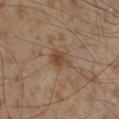notes: no biopsy performed (imaged during a skin exam)
tile lighting: cross-polarized
subject: male, aged approximately 55
body site: the right lower leg
diameter: about 3 mm
acquisition: 15 mm crop, total-body photography
automated metrics: a lesion color around L≈45 a*≈16 b*≈28 in CIELAB and a lesion–skin lightness drop of about 8; a classifier nevus-likeness of about 25/100